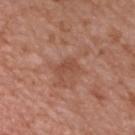This lesion was catalogued during total-body skin photography and was not selected for biopsy. A male subject, aged approximately 50. This is a white-light tile. A 15 mm close-up tile from a total-body photography series done for melanoma screening. From the chest. Automated image analysis of the tile measured a mean CIELAB color near L≈48 a*≈23 b*≈30, roughly 7 lightness units darker than nearby skin, and a normalized lesion–skin contrast near 5.5. And it measured a border-irregularity rating of about 3/10 and a color-variation rating of about 0.5/10. The recorded lesion diameter is about 2.5 mm.